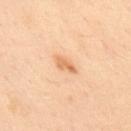Clinical impression:
Part of a total-body skin-imaging series; this lesion was reviewed on a skin check and was not flagged for biopsy.
Context:
Captured under cross-polarized illumination. Automated tile analysis of the lesion measured an average lesion color of about L≈69 a*≈22 b*≈40 (CIELAB) and a lesion-to-skin contrast of about 7 (normalized; higher = more distinct). It also reported a border-irregularity index near 3/10, a within-lesion color-variation index near 2/10, and peripheral color asymmetry of about 1. A male patient, aged approximately 50. A 15 mm crop from a total-body photograph taken for skin-cancer surveillance. From the back.